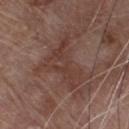workup = total-body-photography surveillance lesion; no biopsy
size = ~6.5 mm (longest diameter)
illumination = white-light
acquisition = 15 mm crop, total-body photography
subject = male, aged 78 to 82
body site = the chest